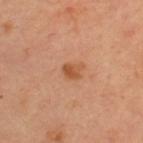Notes:
* biopsy status · total-body-photography surveillance lesion; no biopsy
* site · the upper back
* image · total-body-photography crop, ~15 mm field of view
* patient · female, aged around 45